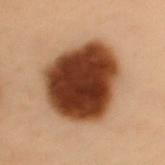Notes:
* lighting: cross-polarized illumination
* site: the back
* lesion size: about 8.5 mm
* image: ~15 mm crop, total-body skin-cancer survey
* subject: female, about 60 years old